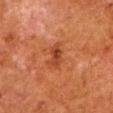notes: catalogued during a skin exam; not biopsied
subject: male, aged 78 to 82
diameter: ~3 mm (longest diameter)
site: the left lower leg
image source: total-body-photography crop, ~15 mm field of view
automated lesion analysis: an average lesion color of about L≈34 a*≈25 b*≈31 (CIELAB), a lesion–skin lightness drop of about 8, and a lesion-to-skin contrast of about 7 (normalized; higher = more distinct); a border-irregularity index near 2.5/10 and a within-lesion color-variation index near 3.5/10
tile lighting: cross-polarized illumination